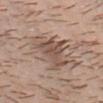follow-up: no biopsy performed (imaged during a skin exam); illumination: white-light; lesion size: ~5 mm (longest diameter); anatomic site: the head or neck; acquisition: 15 mm crop, total-body photography; patient: male, about 30 years old.